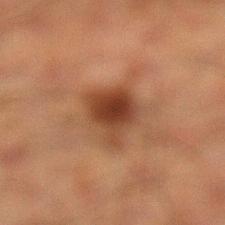Part of a total-body skin-imaging series; this lesion was reviewed on a skin check and was not flagged for biopsy. Cropped from a whole-body photographic skin survey; the tile spans about 15 mm. Approximately 4 mm at its widest. The lesion is on the leg. The tile uses cross-polarized illumination. A male patient, about 50 years old.